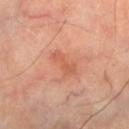The patient is a female approximately 60 years of age. From the right leg. Imaged with cross-polarized lighting. This image is a 15 mm lesion crop taken from a total-body photograph.